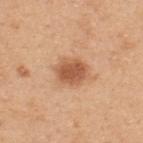Notes:
- follow-up · total-body-photography surveillance lesion; no biopsy
- patient · male, aged approximately 50
- automated lesion analysis · border irregularity of about 2 on a 0–10 scale, internal color variation of about 3 on a 0–10 scale, and a peripheral color-asymmetry measure near 1; a detector confidence of about 100 out of 100 that the crop contains a lesion
- size · ≈3.5 mm
- site · the back
- acquisition · total-body-photography crop, ~15 mm field of view